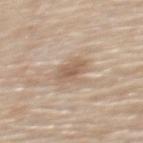Assessment:
The lesion was photographed on a routine skin check and not biopsied; there is no pathology result.
Acquisition and patient details:
Captured under white-light illumination. Approximately 3.5 mm at its widest. A female subject, aged around 65. A 15 mm close-up tile from a total-body photography series done for melanoma screening. The lesion is located on the upper back.